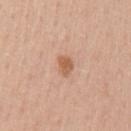{
  "biopsy_status": "not biopsied; imaged during a skin examination",
  "lighting": "white-light",
  "patient": {
    "sex": "female",
    "age_approx": 40
  },
  "lesion_size": {
    "long_diameter_mm_approx": 2.5
  },
  "image": {
    "source": "total-body photography crop",
    "field_of_view_mm": 15
  },
  "site": "arm"
}